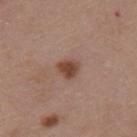Approximately 2.5 mm at its widest. The tile uses white-light illumination. Located on the upper back. A 15 mm close-up tile from a total-body photography series done for melanoma screening. Automated image analysis of the tile measured an area of roughly 4.5 mm² and a shape-asymmetry score of about 0.25 (0 = symmetric). The software also gave a within-lesion color-variation index near 2.5/10 and radial color variation of about 1. A female patient approximately 45 years of age.